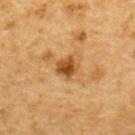follow-up: no biopsy performed (imaged during a skin exam) | site: the back | lesion size: ≈2.5 mm | patient: male, aged 83 or older | image source: total-body-photography crop, ~15 mm field of view.